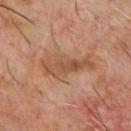biopsy status: no biopsy performed (imaged during a skin exam)
location: the chest
subject: male, roughly 60 years of age
imaging modality: total-body-photography crop, ~15 mm field of view